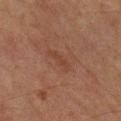Captured during whole-body skin photography for melanoma surveillance; the lesion was not biopsied.
A male patient aged approximately 85 or older.
The lesion is located on the right lower leg.
A close-up tile cropped from a whole-body skin photograph, about 15 mm across.